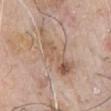The lesion was tiled from a total-body skin photograph and was not biopsied.
The subject is a male aged around 80.
The lesion is located on the chest.
Cropped from a total-body skin-imaging series; the visible field is about 15 mm.